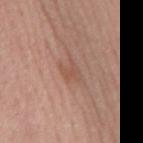* patient · male, in their mid- to late 50s
* lesion size · ≈3 mm
* image source · ~15 mm crop, total-body skin-cancer survey
* anatomic site · the mid back
* image-analysis metrics · a footprint of about 4 mm² and a shape-asymmetry score of about 0.3 (0 = symmetric); a border-irregularity index near 3.5/10, a within-lesion color-variation index near 2/10, and a peripheral color-asymmetry measure near 0.5
* illumination · white-light